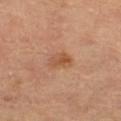Imaged during a routine full-body skin examination; the lesion was not biopsied and no histopathology is available. The lesion's longest dimension is about 2.5 mm. The tile uses cross-polarized illumination. Located on the right thigh. Cropped from a whole-body photographic skin survey; the tile spans about 15 mm. The subject is aged 58–62.